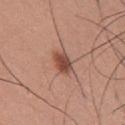site = the upper back | subject = male, in their mid- to late 30s | TBP lesion metrics = a border-irregularity rating of about 2/10 and peripheral color asymmetry of about 1.5 | lesion size = about 3.5 mm | imaging modality = 15 mm crop, total-body photography.